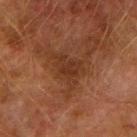notes = catalogued during a skin exam; not biopsied | lighting = cross-polarized | image source = ~15 mm crop, total-body skin-cancer survey | lesion diameter = ~5 mm (longest diameter) | automated lesion analysis = a classifier nevus-likeness of about 0/100 | location = the right upper arm | patient = male, aged 73–77.